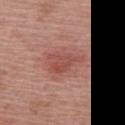Clinical impression:
Imaged during a routine full-body skin examination; the lesion was not biopsied and no histopathology is available.
Image and clinical context:
Automated image analysis of the tile measured an area of roughly 7.5 mm² and two-axis asymmetry of about 0.45. And it measured about 8 CIELAB-L* units darker than the surrounding skin and a normalized border contrast of about 6. The software also gave border irregularity of about 6 on a 0–10 scale and a peripheral color-asymmetry measure near 0.5. Cropped from a whole-body photographic skin survey; the tile spans about 15 mm. Captured under white-light illumination. The recorded lesion diameter is about 4.5 mm. Located on the back. A female patient in their mid- to late 60s.